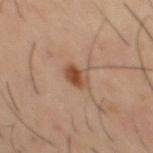{
  "biopsy_status": "not biopsied; imaged during a skin examination",
  "image": {
    "source": "total-body photography crop",
    "field_of_view_mm": 15
  },
  "site": "mid back",
  "patient": {
    "sex": "male",
    "age_approx": 40
  }
}